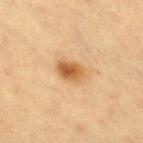No biopsy was performed on this lesion — it was imaged during a full skin examination and was not determined to be concerning.
The lesion's longest dimension is about 3.5 mm.
The tile uses cross-polarized illumination.
On the right thigh.
A female subject roughly 55 years of age.
A roughly 15 mm field-of-view crop from a total-body skin photograph.